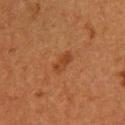{"biopsy_status": "not biopsied; imaged during a skin examination", "site": "back", "automated_metrics": {"area_mm2_approx": 4.0, "eccentricity": 0.85, "shape_asymmetry": 0.25, "cielab_L": 36, "cielab_a": 22, "cielab_b": 33, "vs_skin_darker_L": 6.0, "vs_skin_contrast_norm": 6.0, "nevus_likeness_0_100": 15, "lesion_detection_confidence_0_100": 100}, "lesion_size": {"long_diameter_mm_approx": 3.0}, "image": {"source": "total-body photography crop", "field_of_view_mm": 15}, "patient": {"sex": "female", "age_approx": 40}}